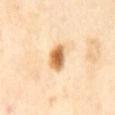TBP lesion metrics = a mean CIELAB color near L≈70 a*≈21 b*≈44 and a normalized border contrast of about 10.5; a classifier nevus-likeness of about 100/100 and a detector confidence of about 100 out of 100 that the crop contains a lesion | acquisition = ~15 mm crop, total-body skin-cancer survey | patient = female, aged around 55 | size = about 3.5 mm | anatomic site = the back | tile lighting = cross-polarized.